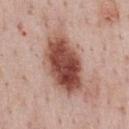Assessment: Recorded during total-body skin imaging; not selected for excision or biopsy. Clinical summary: A 15 mm close-up tile from a total-body photography series done for melanoma screening. Automated tile analysis of the lesion measured a lesion color around L≈49 a*≈24 b*≈27 in CIELAB, about 18 CIELAB-L* units darker than the surrounding skin, and a normalized lesion–skin contrast near 12.5. It also reported border irregularity of about 2 on a 0–10 scale and a within-lesion color-variation index near 7.5/10. It also reported a nevus-likeness score of about 100/100 and a lesion-detection confidence of about 100/100. The lesion is on the front of the torso. A male patient, aged 73–77.